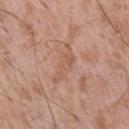Clinical impression:
The lesion was photographed on a routine skin check and not biopsied; there is no pathology result.
Clinical summary:
This is a white-light tile. A roughly 15 mm field-of-view crop from a total-body skin photograph. The subject is a male aged around 50. On the right upper arm.